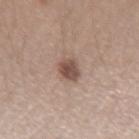Impression:
The lesion was tiled from a total-body skin photograph and was not biopsied.
Background:
This image is a 15 mm lesion crop taken from a total-body photograph. The lesion is on the right forearm. About 2.5 mm across. A female patient, in their mid- to late 40s.